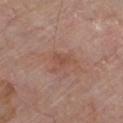<lesion>
  <biopsy_status>not biopsied; imaged during a skin examination</biopsy_status>
  <image>
    <source>total-body photography crop</source>
    <field_of_view_mm>15</field_of_view_mm>
  </image>
  <lesion_size>
    <long_diameter_mm_approx>3.0</long_diameter_mm_approx>
  </lesion_size>
  <patient>
    <sex>male</sex>
    <age_approx>80</age_approx>
  </patient>
  <site>chest</site>
  <lighting>white-light</lighting>
</lesion>